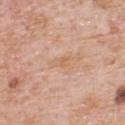Q: Was this lesion biopsied?
A: catalogued during a skin exam; not biopsied
Q: Where on the body is the lesion?
A: the upper back
Q: Who is the patient?
A: male, aged around 80
Q: What kind of image is this?
A: 15 mm crop, total-body photography
Q: Automated lesion metrics?
A: a lesion area of about 3 mm², a shape eccentricity near 0.9, and a shape-asymmetry score of about 0.3 (0 = symmetric); a lesion color around L≈64 a*≈20 b*≈34 in CIELAB, about 6 CIELAB-L* units darker than the surrounding skin, and a lesion-to-skin contrast of about 5.5 (normalized; higher = more distinct); a border-irregularity rating of about 3/10, a within-lesion color-variation index near 1.5/10, and a peripheral color-asymmetry measure near 0.5; a nevus-likeness score of about 0/100